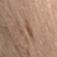biopsy status: no biopsy performed (imaged during a skin exam) | acquisition: 15 mm crop, total-body photography | subject: female, in their mid-40s | TBP lesion metrics: a footprint of about 3 mm², an eccentricity of roughly 0.95, and a shape-asymmetry score of about 0.35 (0 = symmetric); an average lesion color of about L≈47 a*≈17 b*≈26 (CIELAB), about 7 CIELAB-L* units darker than the surrounding skin, and a lesion-to-skin contrast of about 6 (normalized; higher = more distinct); peripheral color asymmetry of about 0; a nevus-likeness score of about 35/100 | lighting: cross-polarized | anatomic site: the arm.